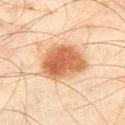<case>
<biopsy_status>not biopsied; imaged during a skin examination</biopsy_status>
<lighting>cross-polarized</lighting>
<lesion_size>
  <long_diameter_mm_approx>5.5</long_diameter_mm_approx>
</lesion_size>
<patient>
  <sex>male</sex>
  <age_approx>45</age_approx>
</patient>
<automated_metrics>
  <area_mm2_approx>20.0</area_mm2_approx>
  <shape_asymmetry>0.2</shape_asymmetry>
  <color_variation_0_10>4.5</color_variation_0_10>
  <peripheral_color_asymmetry>1.5</peripheral_color_asymmetry>
  <nevus_likeness_0_100>100</nevus_likeness_0_100>
  <lesion_detection_confidence_0_100>100</lesion_detection_confidence_0_100>
</automated_metrics>
<image>
  <source>total-body photography crop</source>
  <field_of_view_mm>15</field_of_view_mm>
</image>
<site>left thigh</site>
</case>